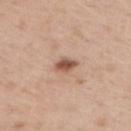| feature | finding |
|---|---|
| follow-up | catalogued during a skin exam; not biopsied |